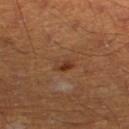Notes:
* biopsy status: total-body-photography surveillance lesion; no biopsy
* subject: male, aged 58–62
* anatomic site: the right lower leg
* illumination: cross-polarized illumination
* size: about 2.5 mm
* automated lesion analysis: border irregularity of about 3 on a 0–10 scale, internal color variation of about 5 on a 0–10 scale, and peripheral color asymmetry of about 1.5; an automated nevus-likeness rating near 80 out of 100 and lesion-presence confidence of about 100/100
* acquisition: ~15 mm crop, total-body skin-cancer survey